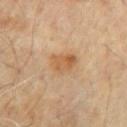biopsy_status: not biopsied; imaged during a skin examination
image:
  source: total-body photography crop
  field_of_view_mm: 15
site: mid back
patient:
  sex: male
  age_approx: 65
lighting: cross-polarized
automated_metrics:
  area_mm2_approx: 7.0
  shape_asymmetry: 0.25
lesion_size:
  long_diameter_mm_approx: 3.5